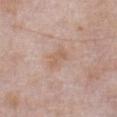body site=the abdomen
tile lighting=white-light
imaging modality=~15 mm tile from a whole-body skin photo
lesion diameter=about 3 mm
automated metrics=an average lesion color of about L≈60 a*≈18 b*≈29 (CIELAB), roughly 6 lightness units darker than nearby skin, and a normalized lesion–skin contrast near 5; a border-irregularity index near 2.5/10; a nevus-likeness score of about 0/100 and lesion-presence confidence of about 100/100
subject=male, roughly 55 years of age